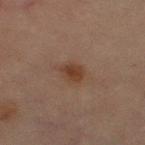Clinical summary: A female patient, in their 70s. Captured under cross-polarized illumination. The lesion is located on the right thigh. The lesion's longest dimension is about 2.5 mm. A lesion tile, about 15 mm wide, cut from a 3D total-body photograph.